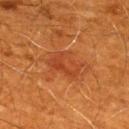Q: What did automated image analysis measure?
A: a mean CIELAB color near L≈42 a*≈31 b*≈39, a lesion–skin lightness drop of about 7, and a normalized lesion–skin contrast near 5.5; border irregularity of about 3.5 on a 0–10 scale, a within-lesion color-variation index near 4.5/10, and peripheral color asymmetry of about 1.5; a classifier nevus-likeness of about 0/100
Q: Where on the body is the lesion?
A: the mid back
Q: What are the patient's age and sex?
A: male, in their 60s
Q: What is the imaging modality?
A: ~15 mm crop, total-body skin-cancer survey